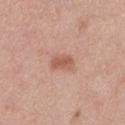Notes:
– notes: catalogued during a skin exam; not biopsied
– imaging modality: 15 mm crop, total-body photography
– subject: female, aged around 55
– anatomic site: the left lower leg
– automated lesion analysis: border irregularity of about 2.5 on a 0–10 scale and a color-variation rating of about 2/10